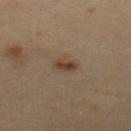{
  "biopsy_status": "not biopsied; imaged during a skin examination",
  "site": "upper back",
  "automated_metrics": {
    "eccentricity": 0.85
  },
  "image": {
    "source": "total-body photography crop",
    "field_of_view_mm": 15
  },
  "patient": {
    "sex": "male",
    "age_approx": 35
  },
  "lighting": "cross-polarized",
  "lesion_size": {
    "long_diameter_mm_approx": 3.0
  }
}